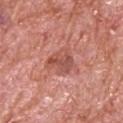This lesion was catalogued during total-body skin photography and was not selected for biopsy.